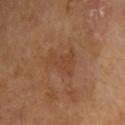A roughly 15 mm field-of-view crop from a total-body skin photograph. The lesion-visualizer software estimated a lesion color around L≈41 a*≈20 b*≈31 in CIELAB and a normalized lesion–skin contrast near 4.5. A male subject aged 63 to 67. Located on the left arm. The recorded lesion diameter is about 4 mm.